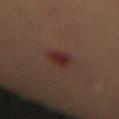The lesion was photographed on a routine skin check and not biopsied; there is no pathology result. About 4 mm across. Automated image analysis of the tile measured a footprint of about 6 mm², a shape eccentricity near 0.9, and a symmetry-axis asymmetry near 0.2. The analysis additionally found an automated nevus-likeness rating near 10 out of 100. This image is a 15 mm lesion crop taken from a total-body photograph. The lesion is on the abdomen. A female patient, aged around 80.